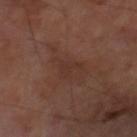image-analysis metrics: an area of roughly 3 mm², a shape eccentricity near 0.8, and a symmetry-axis asymmetry near 0.6; border irregularity of about 6.5 on a 0–10 scale, a color-variation rating of about 0.5/10, and radial color variation of about 0; a classifier nevus-likeness of about 0/100 and a lesion-detection confidence of about 95/100 | patient: male, roughly 70 years of age | image source: 15 mm crop, total-body photography | location: the right thigh.